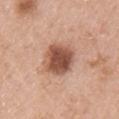Clinical impression: The lesion was photographed on a routine skin check and not biopsied; there is no pathology result. Acquisition and patient details: The lesion is on the chest. The tile uses white-light illumination. A lesion tile, about 15 mm wide, cut from a 3D total-body photograph. A male subject, aged approximately 55.